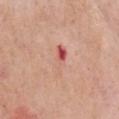The lesion was tiled from a total-body skin photograph and was not biopsied. The lesion is located on the chest. Imaged with white-light lighting. Automated tile analysis of the lesion measured an average lesion color of about L≈60 a*≈27 b*≈29 (CIELAB), about 8 CIELAB-L* units darker than the surrounding skin, and a lesion-to-skin contrast of about 5.5 (normalized; higher = more distinct). The software also gave a border-irregularity index near 4/10, internal color variation of about 10 on a 0–10 scale, and radial color variation of about 6. The software also gave an automated nevus-likeness rating near 0 out of 100. The subject is a male roughly 65 years of age. Longest diameter approximately 4 mm. A region of skin cropped from a whole-body photographic capture, roughly 15 mm wide.